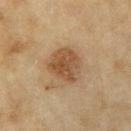Imaged during a routine full-body skin examination; the lesion was not biopsied and no histopathology is available.
Approximately 4.5 mm at its widest.
A lesion tile, about 15 mm wide, cut from a 3D total-body photograph.
A female subject, aged 58 to 62.
Located on the right upper arm.
Captured under cross-polarized illumination.